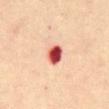Clinical summary: The lesion's longest dimension is about 2.5 mm. A male subject aged 43–47. Cropped from a total-body skin-imaging series; the visible field is about 15 mm. This is a cross-polarized tile. Located on the abdomen.